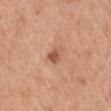Q: Was a biopsy performed?
A: total-body-photography surveillance lesion; no biopsy
Q: Lesion location?
A: the mid back
Q: What is the imaging modality?
A: ~15 mm crop, total-body skin-cancer survey
Q: What are the patient's age and sex?
A: male, about 30 years old
Q: Automated lesion metrics?
A: a border-irregularity index near 3/10, a color-variation rating of about 3/10, and a peripheral color-asymmetry measure near 1; lesion-presence confidence of about 100/100
Q: What is the lesion's diameter?
A: about 2.5 mm